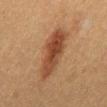Assessment: Part of a total-body skin-imaging series; this lesion was reviewed on a skin check and was not flagged for biopsy. Clinical summary: A male patient aged 48–52. Captured under cross-polarized illumination. A lesion tile, about 15 mm wide, cut from a 3D total-body photograph. The lesion is on the back. Automated image analysis of the tile measured an area of roughly 18 mm², an eccentricity of roughly 0.9, and a symmetry-axis asymmetry near 0.2. It also reported a nevus-likeness score of about 100/100 and a detector confidence of about 100 out of 100 that the crop contains a lesion. About 7 mm across.